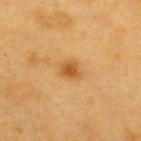| feature | finding |
|---|---|
| follow-up | total-body-photography surveillance lesion; no biopsy |
| patient | male, aged around 60 |
| lighting | cross-polarized illumination |
| image source | total-body-photography crop, ~15 mm field of view |
| body site | the upper back |
| automated metrics | an area of roughly 5 mm², a shape eccentricity near 0.55, and a symmetry-axis asymmetry near 0.3 |
| size | ≈3 mm |